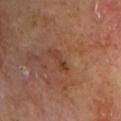{"biopsy_status": "not biopsied; imaged during a skin examination", "site": "chest", "patient": {"sex": "male", "age_approx": 70}, "image": {"source": "total-body photography crop", "field_of_view_mm": 15}, "lesion_size": {"long_diameter_mm_approx": 3.0}}